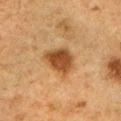Q: Was this lesion biopsied?
A: total-body-photography surveillance lesion; no biopsy
Q: Lesion location?
A: the left upper arm
Q: Lesion size?
A: ≈4 mm
Q: Automated lesion metrics?
A: an area of roughly 11 mm² and an eccentricity of roughly 0.3; a lesion color around L≈39 a*≈19 b*≈33 in CIELAB and a lesion–skin lightness drop of about 12
Q: What are the patient's age and sex?
A: male, aged 48–52
Q: How was this image acquired?
A: ~15 mm crop, total-body skin-cancer survey